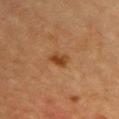The lesion was tiled from a total-body skin photograph and was not biopsied. The total-body-photography lesion software estimated lesion-presence confidence of about 100/100. Longest diameter approximately 2.5 mm. The lesion is located on the chest. A female subject, about 45 years old. A lesion tile, about 15 mm wide, cut from a 3D total-body photograph.